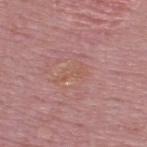No biopsy was performed on this lesion — it was imaged during a full skin examination and was not determined to be concerning.
A lesion tile, about 15 mm wide, cut from a 3D total-body photograph.
The lesion is on the back.
A male subject in their 50s.